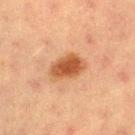Case summary:
• biopsy status — total-body-photography surveillance lesion; no biopsy
• image — ~15 mm crop, total-body skin-cancer survey
• size — ~4.5 mm (longest diameter)
• body site — the left thigh
• patient — female, in their mid- to late 50s